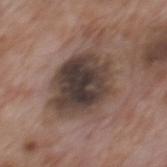Case summary:
• follow-up — no biopsy performed (imaged during a skin exam)
• tile lighting — white-light illumination
• patient — male, approximately 70 years of age
• size — ~8 mm (longest diameter)
• imaging modality — total-body-photography crop, ~15 mm field of view
• image-analysis metrics — an average lesion color of about L≈39 a*≈13 b*≈20 (CIELAB), roughly 14 lightness units darker than nearby skin, and a normalized lesion–skin contrast near 11.5; a nevus-likeness score of about 0/100 and a detector confidence of about 100 out of 100 that the crop contains a lesion
• anatomic site — the mid back